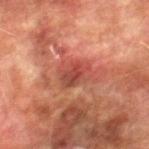The lesion was tiled from a total-body skin photograph and was not biopsied.
The recorded lesion diameter is about 4 mm.
From the leg.
A 15 mm crop from a total-body photograph taken for skin-cancer surveillance.
The lesion-visualizer software estimated peripheral color asymmetry of about 1.5.
A male subject, about 75 years old.